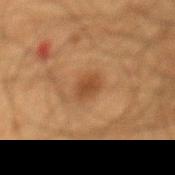{"biopsy_status": "not biopsied; imaged during a skin examination", "lighting": "cross-polarized", "image": {"source": "total-body photography crop", "field_of_view_mm": 15}, "site": "mid back", "patient": {"sex": "male", "age_approx": 65}}